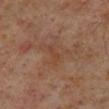biopsy_status: not biopsied; imaged during a skin examination
automated_metrics:
  area_mm2_approx: 2.0
  shape_asymmetry: 0.5
  border_irregularity_0_10: 5.5
  color_variation_0_10: 0.0
patient:
  sex: male
  age_approx: 60
lighting: cross-polarized
image:
  source: total-body photography crop
  field_of_view_mm: 15
site: right lower leg